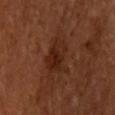workup — imaged on a skin check; not biopsied.